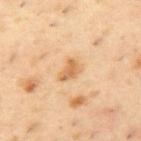Impression:
Part of a total-body skin-imaging series; this lesion was reviewed on a skin check and was not flagged for biopsy.
Background:
A close-up tile cropped from a whole-body skin photograph, about 15 mm across. A male subject aged approximately 55. The lesion is on the upper back. Measured at roughly 3 mm in maximum diameter. Imaged with cross-polarized lighting.